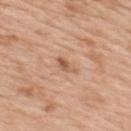follow-up — catalogued during a skin exam; not biopsied | size — ≈2.5 mm | image — ~15 mm tile from a whole-body skin photo | automated metrics — a mean CIELAB color near L≈58 a*≈22 b*≈33 and about 10 CIELAB-L* units darker than the surrounding skin; border irregularity of about 3.5 on a 0–10 scale and internal color variation of about 3 on a 0–10 scale | tile lighting — white-light illumination | location — the upper back | subject — female, in their mid- to late 40s.